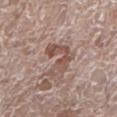This lesion was catalogued during total-body skin photography and was not selected for biopsy.
A roughly 15 mm field-of-view crop from a total-body skin photograph.
On the left lower leg.
Imaged with white-light lighting.
About 4 mm across.
The subject is a female in their mid-80s or older.